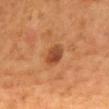Assessment:
No biopsy was performed on this lesion — it was imaged during a full skin examination and was not determined to be concerning.
Acquisition and patient details:
This image is a 15 mm lesion crop taken from a total-body photograph. An algorithmic analysis of the crop reported an area of roughly 5.5 mm², an outline eccentricity of about 0.6 (0 = round, 1 = elongated), and two-axis asymmetry of about 0.15. The analysis additionally found a classifier nevus-likeness of about 70/100 and a lesion-detection confidence of about 100/100. A male patient aged around 55. Approximately 2.5 mm at its widest. The lesion is located on the back. Captured under cross-polarized illumination.